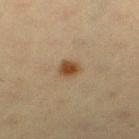Q: Was a biopsy performed?
A: total-body-photography surveillance lesion; no biopsy
Q: Illumination type?
A: cross-polarized
Q: What is the imaging modality?
A: ~15 mm crop, total-body skin-cancer survey
Q: Who is the patient?
A: female, about 40 years old
Q: Automated lesion metrics?
A: a footprint of about 4.5 mm², an eccentricity of roughly 0.55, and two-axis asymmetry of about 0.25; a border-irregularity rating of about 2/10 and internal color variation of about 2.5 on a 0–10 scale; an automated nevus-likeness rating near 100 out of 100 and a detector confidence of about 100 out of 100 that the crop contains a lesion
Q: What is the anatomic site?
A: the leg